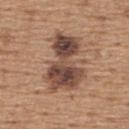This lesion was catalogued during total-body skin photography and was not selected for biopsy.
Measured at roughly 7 mm in maximum diameter.
A male subject, roughly 65 years of age.
Imaged with white-light lighting.
The total-body-photography lesion software estimated an area of roughly 24 mm², an outline eccentricity of about 0.8 (0 = round, 1 = elongated), and two-axis asymmetry of about 0.4. The software also gave a mean CIELAB color near L≈46 a*≈18 b*≈26 and about 14 CIELAB-L* units darker than the surrounding skin. And it measured an automated nevus-likeness rating near 40 out of 100.
A lesion tile, about 15 mm wide, cut from a 3D total-body photograph.
Located on the upper back.